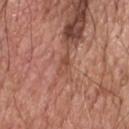Assessment:
Recorded during total-body skin imaging; not selected for excision or biopsy.
Image and clinical context:
A roughly 15 mm field-of-view crop from a total-body skin photograph. Located on the head or neck. Captured under white-light illumination. Approximately 3 mm at its widest. A male subject, in their 80s.